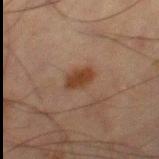Q: Is there a histopathology result?
A: total-body-photography surveillance lesion; no biopsy
Q: Illumination type?
A: cross-polarized illumination
Q: What are the patient's age and sex?
A: male, in their mid-60s
Q: Lesion location?
A: the left thigh
Q: Automated lesion metrics?
A: a footprint of about 5 mm², an eccentricity of roughly 0.75, and a symmetry-axis asymmetry near 0.2; a nevus-likeness score of about 95/100 and lesion-presence confidence of about 100/100
Q: What is the imaging modality?
A: total-body-photography crop, ~15 mm field of view
Q: Lesion size?
A: ≈3 mm